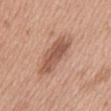Clinical impression:
No biopsy was performed on this lesion — it was imaged during a full skin examination and was not determined to be concerning.
Context:
The lesion is on the abdomen. A roughly 15 mm field-of-view crop from a total-body skin photograph. Captured under white-light illumination. The patient is a male approximately 75 years of age.